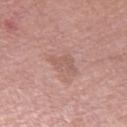biopsy_status: not biopsied; imaged during a skin examination
patient:
  sex: male
  age_approx: 80
lighting: white-light
lesion_size:
  long_diameter_mm_approx: 3.5
image:
  source: total-body photography crop
  field_of_view_mm: 15
site: right thigh